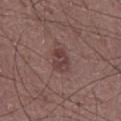Imaged during a routine full-body skin examination; the lesion was not biopsied and no histopathology is available.
On the front of the torso.
A 15 mm crop from a total-body photograph taken for skin-cancer surveillance.
A male patient about 60 years old.
Captured under white-light illumination.